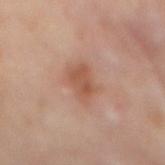Q: Was this lesion biopsied?
A: imaged on a skin check; not biopsied
Q: Where on the body is the lesion?
A: the mid back
Q: What did automated image analysis measure?
A: an area of roughly 9 mm², an outline eccentricity of about 0.85 (0 = round, 1 = elongated), and a symmetry-axis asymmetry near 0.25; internal color variation of about 2.5 on a 0–10 scale and a peripheral color-asymmetry measure near 1
Q: What is the imaging modality?
A: 15 mm crop, total-body photography
Q: What are the patient's age and sex?
A: female, in their 60s
Q: What lighting was used for the tile?
A: cross-polarized